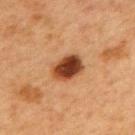• workup: no biopsy performed (imaged during a skin exam)
• lighting: cross-polarized
• imaging modality: 15 mm crop, total-body photography
• automated lesion analysis: an eccentricity of roughly 0.7 and two-axis asymmetry of about 0.2; border irregularity of about 1.5 on a 0–10 scale, a color-variation rating of about 6.5/10, and a peripheral color-asymmetry measure near 2; a classifier nevus-likeness of about 100/100 and a detector confidence of about 100 out of 100 that the crop contains a lesion
• site: the mid back
• lesion size: about 4 mm
• patient: female, about 40 years old